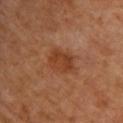automated lesion analysis: an automated nevus-likeness rating near 20 out of 100; tile lighting: cross-polarized illumination; size: ~4 mm (longest diameter); patient: female, aged approximately 65; acquisition: ~15 mm crop, total-body skin-cancer survey; anatomic site: the back.